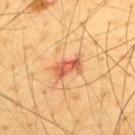The lesion was tiled from a total-body skin photograph and was not biopsied.
Imaged with cross-polarized lighting.
The recorded lesion diameter is about 3.5 mm.
Cropped from a total-body skin-imaging series; the visible field is about 15 mm.
The lesion is located on the upper back.
The subject is a male in their mid- to late 60s.